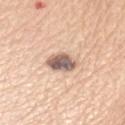Impression: Part of a total-body skin-imaging series; this lesion was reviewed on a skin check and was not flagged for biopsy. Clinical summary: The lesion's longest dimension is about 3.5 mm. The lesion is on the arm. A female patient aged around 60. Captured under white-light illumination. A region of skin cropped from a whole-body photographic capture, roughly 15 mm wide.